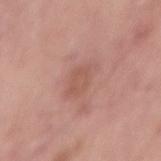The lesion was tiled from a total-body skin photograph and was not biopsied.
The patient is a male aged around 55.
A region of skin cropped from a whole-body photographic capture, roughly 15 mm wide.
Automated image analysis of the tile measured a lesion color around L≈56 a*≈22 b*≈27 in CIELAB and a normalized border contrast of about 5.
Longest diameter approximately 3.5 mm.
From the lower back.